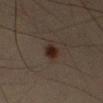Findings:
• notes — catalogued during a skin exam; not biopsied
• automated lesion analysis — a lesion area of about 5 mm², an outline eccentricity of about 0.55 (0 = round, 1 = elongated), and a shape-asymmetry score of about 0.2 (0 = symmetric); a classifier nevus-likeness of about 100/100 and a detector confidence of about 100 out of 100 that the crop contains a lesion
• lesion diameter — ~2.5 mm (longest diameter)
• location — the right upper arm
• lighting — cross-polarized
• subject — male, roughly 40 years of age
• imaging modality — 15 mm crop, total-body photography